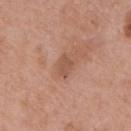notes: imaged on a skin check; not biopsied | automated lesion analysis: a shape-asymmetry score of about 0.35 (0 = symmetric); a border-irregularity index near 3/10, internal color variation of about 1.5 on a 0–10 scale, and radial color variation of about 0.5; a classifier nevus-likeness of about 0/100 and lesion-presence confidence of about 100/100 | tile lighting: white-light illumination | imaging modality: total-body-photography crop, ~15 mm field of view | subject: male, approximately 70 years of age | location: the back | size: ~2.5 mm (longest diameter).